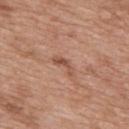Findings:
• follow-up: total-body-photography surveillance lesion; no biopsy
• subject: male, aged 48 to 52
• tile lighting: white-light
• automated metrics: a footprint of about 3 mm², an eccentricity of roughly 0.9, and two-axis asymmetry of about 0.6
• lesion size: about 3 mm
• image source: ~15 mm tile from a whole-body skin photo
• site: the mid back